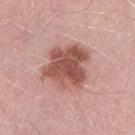Captured during whole-body skin photography for melanoma surveillance; the lesion was not biopsied. The total-body-photography lesion software estimated a border-irregularity rating of about 2.5/10 and a color-variation rating of about 5.5/10. A male subject aged 53 to 57. Cropped from a whole-body photographic skin survey; the tile spans about 15 mm. From the left thigh. About 6 mm across.